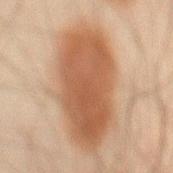Notes:
• follow-up: total-body-photography surveillance lesion; no biopsy